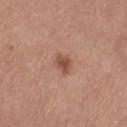Recorded during total-body skin imaging; not selected for excision or biopsy. On the abdomen. Automated image analysis of the tile measured a footprint of about 4 mm². It also reported an average lesion color of about L≈51 a*≈23 b*≈29 (CIELAB) and a normalized lesion–skin contrast near 7.5. It also reported a border-irregularity rating of about 2.5/10, a color-variation rating of about 2/10, and a peripheral color-asymmetry measure near 0.5. A female subject, aged around 75. A roughly 15 mm field-of-view crop from a total-body skin photograph. Captured under white-light illumination. The recorded lesion diameter is about 2.5 mm.